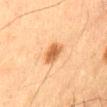<lesion>
<biopsy_status>not biopsied; imaged during a skin examination</biopsy_status>
<image>
  <source>total-body photography crop</source>
  <field_of_view_mm>15</field_of_view_mm>
</image>
<lesion_size>
  <long_diameter_mm_approx>3.0</long_diameter_mm_approx>
</lesion_size>
<automated_metrics>
  <border_irregularity_0_10>2.0</border_irregularity_0_10>
  <color_variation_0_10>2.5</color_variation_0_10>
</automated_metrics>
<patient>
  <sex>male</sex>
  <age_approx>65</age_approx>
</patient>
<lighting>cross-polarized</lighting>
<site>back</site>
</lesion>